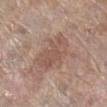Imaged during a routine full-body skin examination; the lesion was not biopsied and no histopathology is available. Cropped from a total-body skin-imaging series; the visible field is about 15 mm. The lesion is located on the leg. The subject is a female approximately 85 years of age or older. The tile uses white-light illumination.